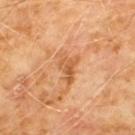Part of a total-body skin-imaging series; this lesion was reviewed on a skin check and was not flagged for biopsy. Longest diameter approximately 3.5 mm. From the chest. Captured under cross-polarized illumination. A male patient aged around 60. A 15 mm crop from a total-body photograph taken for skin-cancer surveillance. Automated image analysis of the tile measured an area of roughly 6 mm², an outline eccentricity of about 0.75 (0 = round, 1 = elongated), and a symmetry-axis asymmetry near 0.45. The software also gave a mean CIELAB color near L≈56 a*≈25 b*≈40, roughly 9 lightness units darker than nearby skin, and a normalized border contrast of about 6.5. The analysis additionally found a border-irregularity rating of about 5.5/10, a color-variation rating of about 4/10, and radial color variation of about 1.5.